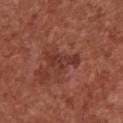{
  "biopsy_status": "not biopsied; imaged during a skin examination",
  "image": {
    "source": "total-body photography crop",
    "field_of_view_mm": 15
  },
  "lesion_size": {
    "long_diameter_mm_approx": 4.5
  },
  "site": "chest",
  "patient": {
    "sex": "female",
    "age_approx": 75
  },
  "lighting": "white-light",
  "automated_metrics": {
    "border_irregularity_0_10": 5.5,
    "peripheral_color_asymmetry": 1.0,
    "nevus_likeness_0_100": 0,
    "lesion_detection_confidence_0_100": 100
  }
}